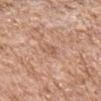{
  "site": "arm",
  "patient": {
    "sex": "male",
    "age_approx": 65
  },
  "lighting": "white-light",
  "image": {
    "source": "total-body photography crop",
    "field_of_view_mm": 15
  }
}